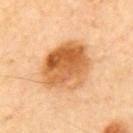workup = imaged on a skin check; not biopsied | illumination = cross-polarized | lesion size = ~6.5 mm (longest diameter) | anatomic site = the back | image source = total-body-photography crop, ~15 mm field of view.